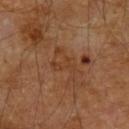Q: Was this lesion biopsied?
A: imaged on a skin check; not biopsied
Q: Illumination type?
A: cross-polarized illumination
Q: What kind of image is this?
A: ~15 mm crop, total-body skin-cancer survey
Q: What did automated image analysis measure?
A: a lesion area of about 7.5 mm², an eccentricity of roughly 0.8, and a shape-asymmetry score of about 0.45 (0 = symmetric); an automated nevus-likeness rating near 0 out of 100 and a detector confidence of about 90 out of 100 that the crop contains a lesion
Q: How large is the lesion?
A: ≈4.5 mm
Q: Patient demographics?
A: male, in their 60s
Q: What is the anatomic site?
A: the left forearm